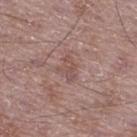  biopsy_status: not biopsied; imaged during a skin examination
  automated_metrics:
    eccentricity: 0.75
    shape_asymmetry: 0.3
    cielab_L: 50
    cielab_a: 20
    cielab_b: 22
    vs_skin_darker_L: 7.0
    vs_skin_contrast_norm: 5.0
    border_irregularity_0_10: 4.0
    color_variation_0_10: 2.0
    peripheral_color_asymmetry: 0.5
    nevus_likeness_0_100: 0
  lesion_size:
    long_diameter_mm_approx: 2.5
  site: right thigh
  patient:
    sex: male
    age_approx: 50
  image:
    source: total-body photography crop
    field_of_view_mm: 15